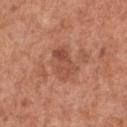follow-up = no biopsy performed (imaged during a skin exam) | diameter = about 4 mm | site = the chest | image = 15 mm crop, total-body photography | subject = male, in their mid- to late 60s.